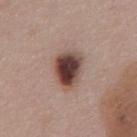{"biopsy_status": "not biopsied; imaged during a skin examination", "image": {"source": "total-body photography crop", "field_of_view_mm": 15}, "patient": {"sex": "male", "age_approx": 55}, "lighting": "white-light", "site": "front of the torso", "lesion_size": {"long_diameter_mm_approx": 4.0}}